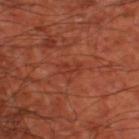This lesion was catalogued during total-body skin photography and was not selected for biopsy. From the left thigh. The patient is aged approximately 65. This is a cross-polarized tile. Measured at roughly 2.5 mm in maximum diameter. Cropped from a total-body skin-imaging series; the visible field is about 15 mm. Automated tile analysis of the lesion measured an average lesion color of about L≈36 a*≈29 b*≈33 (CIELAB), about 5 CIELAB-L* units darker than the surrounding skin, and a normalized border contrast of about 4.5. And it measured border irregularity of about 4.5 on a 0–10 scale and radial color variation of about 0.5. The software also gave a classifier nevus-likeness of about 0/100.